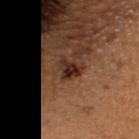The tile uses cross-polarized illumination.
A female patient aged 38–42.
A 15 mm close-up tile from a total-body photography series done for melanoma screening.
On the head or neck.